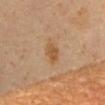Q: Was this lesion biopsied?
A: total-body-photography surveillance lesion; no biopsy
Q: What are the patient's age and sex?
A: male, aged approximately 60
Q: What is the anatomic site?
A: the mid back
Q: How was this image acquired?
A: ~15 mm tile from a whole-body skin photo